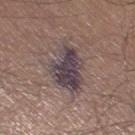The tile uses white-light illumination. The total-body-photography lesion software estimated an area of roughly 19 mm², an eccentricity of roughly 0.8, and two-axis asymmetry of about 0.25. It also reported a mean CIELAB color near L≈42 a*≈13 b*≈12, roughly 11 lightness units darker than nearby skin, and a normalized lesion–skin contrast near 11.5. The software also gave a nevus-likeness score of about 0/100 and lesion-presence confidence of about 95/100. A male patient approximately 35 years of age. On the right thigh. Approximately 7 mm at its widest. A region of skin cropped from a whole-body photographic capture, roughly 15 mm wide.